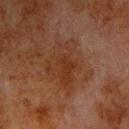Part of a total-body skin-imaging series; this lesion was reviewed on a skin check and was not flagged for biopsy.
Captured under cross-polarized illumination.
A male patient, in their 80s.
An algorithmic analysis of the crop reported a nevus-likeness score of about 0/100 and a detector confidence of about 100 out of 100 that the crop contains a lesion.
A close-up tile cropped from a whole-body skin photograph, about 15 mm across.
Longest diameter approximately 6 mm.
Located on the front of the torso.